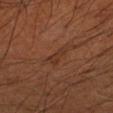notes=no biopsy performed (imaged during a skin exam)
diameter=~3 mm (longest diameter)
subject=male, approximately 60 years of age
image source=~15 mm tile from a whole-body skin photo
location=the left upper arm